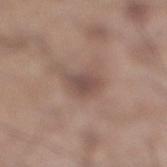The lesion was photographed on a routine skin check and not biopsied; there is no pathology result.
This image is a 15 mm lesion crop taken from a total-body photograph.
A male patient, roughly 60 years of age.
Located on the right lower leg.